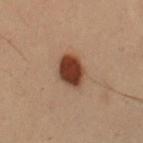Impression: The lesion was tiled from a total-body skin photograph and was not biopsied. Context: The lesion is on the arm. Imaged with cross-polarized lighting. A roughly 15 mm field-of-view crop from a total-body skin photograph. Approximately 4 mm at its widest. Automated image analysis of the tile measured a lesion area of about 10 mm² and a symmetry-axis asymmetry near 0.15. It also reported an average lesion color of about L≈32 a*≈18 b*≈25 (CIELAB), about 14 CIELAB-L* units darker than the surrounding skin, and a normalized lesion–skin contrast near 13. The patient is a male aged 53–57.